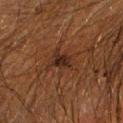biopsy status: catalogued during a skin exam; not biopsied | image source: ~15 mm tile from a whole-body skin photo | subject: male, aged 58–62 | site: the right forearm.